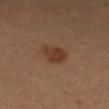Background: Longest diameter approximately 3.5 mm. Cropped from a whole-body photographic skin survey; the tile spans about 15 mm. The tile uses cross-polarized illumination. Automated image analysis of the tile measured a border-irregularity rating of about 2/10, internal color variation of about 2.5 on a 0–10 scale, and radial color variation of about 1. It also reported an automated nevus-likeness rating near 95 out of 100 and lesion-presence confidence of about 100/100. The lesion is on the left arm. A female patient, aged 38 to 42.